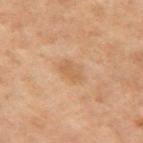Assessment:
Imaged during a routine full-body skin examination; the lesion was not biopsied and no histopathology is available.
Context:
The lesion's longest dimension is about 3 mm. Cropped from a whole-body photographic skin survey; the tile spans about 15 mm. On the right upper arm. This is a cross-polarized tile. Automated tile analysis of the lesion measured a footprint of about 6.5 mm², an outline eccentricity of about 0.6 (0 = round, 1 = elongated), and a shape-asymmetry score of about 0.25 (0 = symmetric). And it measured an average lesion color of about L≈45 a*≈15 b*≈28 (CIELAB), about 5 CIELAB-L* units darker than the surrounding skin, and a normalized border contrast of about 4.5. And it measured a within-lesion color-variation index near 2/10. The analysis additionally found an automated nevus-likeness rating near 5 out of 100 and lesion-presence confidence of about 100/100. A male patient aged approximately 70.